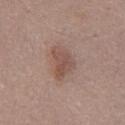The lesion was photographed on a routine skin check and not biopsied; there is no pathology result.
The patient is a female aged 38 to 42.
From the mid back.
Captured under white-light illumination.
Automated tile analysis of the lesion measured an average lesion color of about L≈52 a*≈18 b*≈24 (CIELAB), a lesion–skin lightness drop of about 8, and a normalized border contrast of about 6.
Cropped from a total-body skin-imaging series; the visible field is about 15 mm.